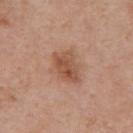<tbp_lesion>
<biopsy_status>not biopsied; imaged during a skin examination</biopsy_status>
<lesion_size>
  <long_diameter_mm_approx>4.0</long_diameter_mm_approx>
</lesion_size>
<image>
  <source>total-body photography crop</source>
  <field_of_view_mm>15</field_of_view_mm>
</image>
<site>upper back</site>
<automated_metrics>
  <border_irregularity_0_10>3.5</border_irregularity_0_10>
  <color_variation_0_10>4.0</color_variation_0_10>
  <peripheral_color_asymmetry>1.5</peripheral_color_asymmetry>
</automated_metrics>
<lighting>white-light</lighting>
<patient>
  <sex>male</sex>
  <age_approx>55</age_approx>
</patient>
</tbp_lesion>